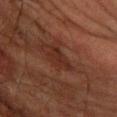Captured during whole-body skin photography for melanoma surveillance; the lesion was not biopsied.
Cropped from a whole-body photographic skin survey; the tile spans about 15 mm.
Imaged with cross-polarized lighting.
Located on the arm.
A male patient aged approximately 65.
The lesion-visualizer software estimated an area of roughly 5.5 mm², a shape eccentricity near 0.8, and a symmetry-axis asymmetry near 0.4. And it measured a border-irregularity rating of about 4.5/10, a within-lesion color-variation index near 2/10, and peripheral color asymmetry of about 0.5.
Measured at roughly 3.5 mm in maximum diameter.